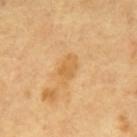Findings:
• workup — catalogued during a skin exam; not biopsied
• image source — ~15 mm tile from a whole-body skin photo
• lesion size — ~3.5 mm (longest diameter)
• location — the mid back
• patient — male, aged around 65
• illumination — cross-polarized illumination
• TBP lesion metrics — an area of roughly 5 mm² and an eccentricity of roughly 0.8; a normalized border contrast of about 5.5; a border-irregularity index near 3/10, internal color variation of about 2 on a 0–10 scale, and radial color variation of about 0.5; a classifier nevus-likeness of about 0/100